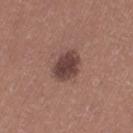<case>
  <biopsy_status>not biopsied; imaged during a skin examination</biopsy_status>
  <image>
    <source>total-body photography crop</source>
    <field_of_view_mm>15</field_of_view_mm>
  </image>
  <lesion_size>
    <long_diameter_mm_approx>3.5</long_diameter_mm_approx>
  </lesion_size>
  <patient>
    <sex>female</sex>
    <age_approx>40</age_approx>
  </patient>
  <site>left thigh</site>
</case>